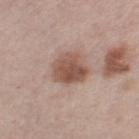{
  "biopsy_status": "not biopsied; imaged during a skin examination",
  "patient": {
    "sex": "male",
    "age_approx": 65
  },
  "automated_metrics": {
    "border_irregularity_0_10": 2.0,
    "color_variation_0_10": 5.0,
    "nevus_likeness_0_100": 85,
    "lesion_detection_confidence_0_100": 100
  },
  "site": "left thigh",
  "image": {
    "source": "total-body photography crop",
    "field_of_view_mm": 15
  }
}